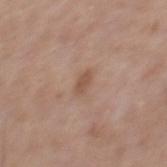Case summary:
* biopsy status — no biopsy performed (imaged during a skin exam)
* location — the mid back
* automated metrics — a mean CIELAB color near L≈52 a*≈19 b*≈28 and a normalized border contrast of about 6.5; peripheral color asymmetry of about 0
* lesion size — ~2.5 mm (longest diameter)
* subject — male, in their mid-50s
* image source — 15 mm crop, total-body photography